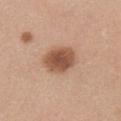The lesion was tiled from a total-body skin photograph and was not biopsied. A 15 mm close-up extracted from a 3D total-body photography capture. Imaged with white-light lighting. A female subject aged 18 to 22. The lesion is on the chest. The total-body-photography lesion software estimated a mean CIELAB color near L≈53 a*≈21 b*≈31 and roughly 14 lightness units darker than nearby skin. The software also gave a nevus-likeness score of about 95/100. The lesion's longest dimension is about 4.5 mm.